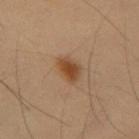Assessment:
Captured during whole-body skin photography for melanoma surveillance; the lesion was not biopsied.
Image and clinical context:
The total-body-photography lesion software estimated border irregularity of about 2.5 on a 0–10 scale, a color-variation rating of about 3/10, and a peripheral color-asymmetry measure near 1. The lesion is located on the mid back. A male subject roughly 55 years of age. Measured at roughly 3 mm in maximum diameter. A lesion tile, about 15 mm wide, cut from a 3D total-body photograph.